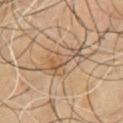Acquisition and patient details:
Imaged with cross-polarized lighting. A roughly 15 mm field-of-view crop from a total-body skin photograph. The lesion is on the chest. A male patient aged 63–67.